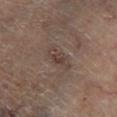No biopsy was performed on this lesion — it was imaged during a full skin examination and was not determined to be concerning. The lesion is located on the right lower leg. Cropped from a total-body skin-imaging series; the visible field is about 15 mm. Captured under cross-polarized illumination. A male patient in their mid- to late 60s.